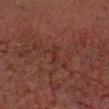Q: Was this lesion biopsied?
A: total-body-photography surveillance lesion; no biopsy
Q: How was the tile lit?
A: cross-polarized illumination
Q: What kind of image is this?
A: 15 mm crop, total-body photography
Q: What are the patient's age and sex?
A: male, aged approximately 60
Q: What is the lesion's diameter?
A: ≈3 mm
Q: What did automated image analysis measure?
A: an eccentricity of roughly 0.95 and a shape-asymmetry score of about 0.35 (0 = symmetric); a mean CIELAB color near L≈22 a*≈20 b*≈19, a lesion–skin lightness drop of about 4, and a normalized border contrast of about 4.5; border irregularity of about 4.5 on a 0–10 scale
Q: Lesion location?
A: the head or neck